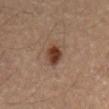workup: catalogued during a skin exam; not biopsied
acquisition: ~15 mm tile from a whole-body skin photo
patient: male, aged around 55
diameter: ~3.5 mm (longest diameter)
illumination: cross-polarized illumination
anatomic site: the abdomen